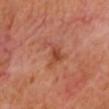Impression:
Imaged during a routine full-body skin examination; the lesion was not biopsied and no histopathology is available.
Background:
The lesion-visualizer software estimated an area of roughly 6.5 mm², an eccentricity of roughly 0.9, and a shape-asymmetry score of about 0.6 (0 = symmetric). It also reported border irregularity of about 7.5 on a 0–10 scale, a color-variation rating of about 4/10, and radial color variation of about 1.5. It also reported a classifier nevus-likeness of about 0/100 and a lesion-detection confidence of about 100/100. The lesion is on the head or neck. The lesion's longest dimension is about 5 mm. A close-up tile cropped from a whole-body skin photograph, about 15 mm across. Captured under cross-polarized illumination.